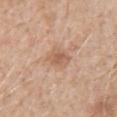<case>
  <biopsy_status>not biopsied; imaged during a skin examination</biopsy_status>
  <patient>
    <sex>male</sex>
    <age_approx>60</age_approx>
  </patient>
  <image>
    <source>total-body photography crop</source>
    <field_of_view_mm>15</field_of_view_mm>
  </image>
  <lesion_size>
    <long_diameter_mm_approx>3.0</long_diameter_mm_approx>
  </lesion_size>
  <lighting>white-light</lighting>
  <site>arm</site>
</case>